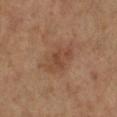Q: Is there a histopathology result?
A: total-body-photography surveillance lesion; no biopsy
Q: Lesion location?
A: the leg
Q: Who is the patient?
A: female, in their mid-60s
Q: Automated lesion metrics?
A: a lesion area of about 13 mm², a shape eccentricity near 0.7, and a shape-asymmetry score of about 0.25 (0 = symmetric); an average lesion color of about L≈45 a*≈19 b*≈29 (CIELAB), about 7 CIELAB-L* units darker than the surrounding skin, and a normalized border contrast of about 5.5; a classifier nevus-likeness of about 10/100 and a lesion-detection confidence of about 100/100
Q: How large is the lesion?
A: ~5 mm (longest diameter)
Q: What kind of image is this?
A: 15 mm crop, total-body photography